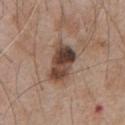Context:
A male patient in their mid- to late 60s. An algorithmic analysis of the crop reported a lesion area of about 12 mm², a shape eccentricity near 0.8, and a shape-asymmetry score of about 0.2 (0 = symmetric). It also reported a nevus-likeness score of about 40/100 and lesion-presence confidence of about 100/100. Captured under white-light illumination. The lesion is located on the chest. A lesion tile, about 15 mm wide, cut from a 3D total-body photograph.